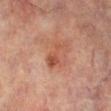<tbp_lesion>
<biopsy_status>not biopsied; imaged during a skin examination</biopsy_status>
<automated_metrics>
  <border_irregularity_0_10>7.0</border_irregularity_0_10>
  <color_variation_0_10>2.0</color_variation_0_10>
  <peripheral_color_asymmetry>0.5</peripheral_color_asymmetry>
  <lesion_detection_confidence_0_100>100</lesion_detection_confidence_0_100>
</automated_metrics>
<lesion_size>
  <long_diameter_mm_approx>4.0</long_diameter_mm_approx>
</lesion_size>
<patient>
  <sex>female</sex>
  <age_approx>70</age_approx>
</patient>
<site>right lower leg</site>
<image>
  <source>total-body photography crop</source>
  <field_of_view_mm>15</field_of_view_mm>
</image>
<lighting>cross-polarized</lighting>
</tbp_lesion>